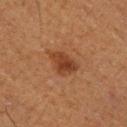Q: Was a biopsy performed?
A: catalogued during a skin exam; not biopsied
Q: Illumination type?
A: cross-polarized
Q: How was this image acquired?
A: 15 mm crop, total-body photography
Q: Lesion size?
A: about 4 mm
Q: Lesion location?
A: the leg
Q: Who is the patient?
A: male, approximately 75 years of age